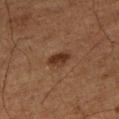Q: Was a biopsy performed?
A: no biopsy performed (imaged during a skin exam)
Q: What is the imaging modality?
A: ~15 mm tile from a whole-body skin photo
Q: What is the anatomic site?
A: the leg
Q: How was the tile lit?
A: cross-polarized
Q: What are the patient's age and sex?
A: male, in their mid- to late 70s